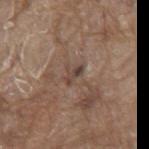Clinical impression:
Imaged during a routine full-body skin examination; the lesion was not biopsied and no histopathology is available.
Image and clinical context:
The lesion's longest dimension is about 2.5 mm. A 15 mm close-up tile from a total-body photography series done for melanoma screening. Imaged with white-light lighting. The lesion-visualizer software estimated a footprint of about 2.5 mm² and an eccentricity of roughly 0.95. And it measured a lesion color around L≈42 a*≈15 b*≈23 in CIELAB, about 9 CIELAB-L* units darker than the surrounding skin, and a lesion-to-skin contrast of about 7.5 (normalized; higher = more distinct). And it measured an automated nevus-likeness rating near 0 out of 100. The lesion is on the mid back. The subject is a male aged approximately 80.